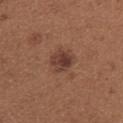<case>
  <biopsy_status>not biopsied; imaged during a skin examination</biopsy_status>
  <image>
    <source>total-body photography crop</source>
    <field_of_view_mm>15</field_of_view_mm>
  </image>
  <patient>
    <sex>female</sex>
    <age_approx>45</age_approx>
  </patient>
  <site>right upper arm</site>
  <automated_metrics>
    <border_irregularity_0_10>2.0</border_irregularity_0_10>
    <color_variation_0_10>5.0</color_variation_0_10>
    <peripheral_color_asymmetry>2.0</peripheral_color_asymmetry>
    <lesion_detection_confidence_0_100>100</lesion_detection_confidence_0_100>
  </automated_metrics>
  <lighting>white-light</lighting>
</case>